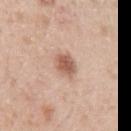  biopsy_status: not biopsied; imaged during a skin examination
  automated_metrics:
    color_variation_0_10: 3.5
    peripheral_color_asymmetry: 1.0
  lighting: white-light
  lesion_size:
    long_diameter_mm_approx: 3.0
  image:
    source: total-body photography crop
    field_of_view_mm: 15
  site: left upper arm
  patient:
    sex: male
    age_approx: 40